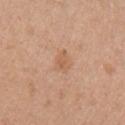workup — no biopsy performed (imaged during a skin exam) | automated lesion analysis — an area of roughly 4.5 mm², a shape eccentricity near 0.65, and two-axis asymmetry of about 0.3; a lesion color around L≈60 a*≈20 b*≈34 in CIELAB | size — ≈3 mm | lighting — white-light | subject — female, aged 63 to 67 | location — the left upper arm | acquisition — ~15 mm tile from a whole-body skin photo.